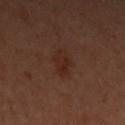  biopsy_status: not biopsied; imaged during a skin examination
  lighting: cross-polarized
  automated_metrics:
    eccentricity: 0.7
    cielab_L: 26
    cielab_a: 19
    cielab_b: 25
    vs_skin_darker_L: 5.0
    lesion_detection_confidence_0_100: 100
  patient:
    sex: female
    age_approx: 30
  site: left upper arm
  image:
    source: total-body photography crop
    field_of_view_mm: 15